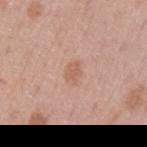The lesion was photographed on a routine skin check and not biopsied; there is no pathology result. On the left upper arm. Captured under white-light illumination. The recorded lesion diameter is about 2.5 mm. The subject is a male in their mid- to late 40s. A roughly 15 mm field-of-view crop from a total-body skin photograph. The total-body-photography lesion software estimated about 7 CIELAB-L* units darker than the surrounding skin and a lesion-to-skin contrast of about 5.5 (normalized; higher = more distinct). The software also gave a classifier nevus-likeness of about 15/100 and a lesion-detection confidence of about 100/100.